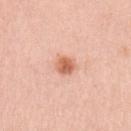Q: Was a biopsy performed?
A: imaged on a skin check; not biopsied
Q: Where on the body is the lesion?
A: the left upper arm
Q: What lighting was used for the tile?
A: white-light illumination
Q: What are the patient's age and sex?
A: female, aged around 45
Q: What is the imaging modality?
A: 15 mm crop, total-body photography
Q: Automated lesion metrics?
A: an automated nevus-likeness rating near 95 out of 100 and lesion-presence confidence of about 100/100
Q: Lesion size?
A: about 2.5 mm